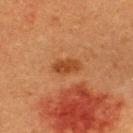Findings:
- biopsy status — no biopsy performed (imaged during a skin exam)
- acquisition — 15 mm crop, total-body photography
- lesion diameter — ~3.5 mm (longest diameter)
- lighting — cross-polarized
- anatomic site — the upper back
- subject — male, approximately 40 years of age